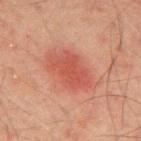Clinical impression:
The lesion was tiled from a total-body skin photograph and was not biopsied.
Clinical summary:
The recorded lesion diameter is about 5.5 mm. On the back. A male patient, aged 43–47. A close-up tile cropped from a whole-body skin photograph, about 15 mm across. Imaged with cross-polarized lighting.